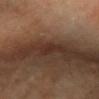| field | value |
|---|---|
| notes | total-body-photography surveillance lesion; no biopsy |
| site | the right forearm |
| image source | ~15 mm tile from a whole-body skin photo |
| automated lesion analysis | a footprint of about 2.5 mm², an eccentricity of roughly 0.9, and two-axis asymmetry of about 0.25; an average lesion color of about L≈29 a*≈18 b*≈23 (CIELAB) and a normalized lesion–skin contrast near 6 |
| subject | female, aged 48 to 52 |